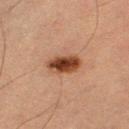The lesion was photographed on a routine skin check and not biopsied; there is no pathology result. On the left thigh. A female patient aged 58–62. Cropped from a whole-body photographic skin survey; the tile spans about 15 mm. The tile uses cross-polarized illumination. The lesion's longest dimension is about 4 mm.